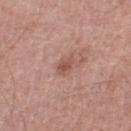{"biopsy_status": "not biopsied; imaged during a skin examination", "image": {"source": "total-body photography crop", "field_of_view_mm": 15}, "lighting": "white-light", "lesion_size": {"long_diameter_mm_approx": 2.5}, "site": "right lower leg", "patient": {"sex": "male", "age_approx": 60}}